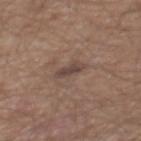The lesion was photographed on a routine skin check and not biopsied; there is no pathology result. The subject is a male aged 63–67. A 15 mm crop from a total-body photograph taken for skin-cancer surveillance. The recorded lesion diameter is about 3 mm. On the back.